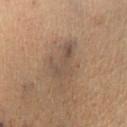follow-up: total-body-photography surveillance lesion; no biopsy | patient: male, aged 48 to 52 | lighting: cross-polarized | site: the right lower leg | diameter: about 5 mm | image: total-body-photography crop, ~15 mm field of view.